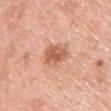Part of a total-body skin-imaging series; this lesion was reviewed on a skin check and was not flagged for biopsy. Longest diameter approximately 3.5 mm. From the chest. Captured under white-light illumination. A region of skin cropped from a whole-body photographic capture, roughly 15 mm wide. The patient is a male aged around 60.